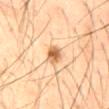A 15 mm close-up tile from a total-body photography series done for melanoma screening. The lesion-visualizer software estimated an area of roughly 4 mm² and a shape eccentricity near 0.75. The software also gave a nevus-likeness score of about 95/100 and a detector confidence of about 100 out of 100 that the crop contains a lesion. This is a cross-polarized tile. A male patient aged approximately 45. On the mid back. About 2.5 mm across.